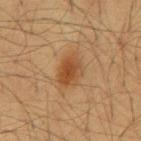Assessment: Imaged during a routine full-body skin examination; the lesion was not biopsied and no histopathology is available. Context: Approximately 4 mm at its widest. Automated tile analysis of the lesion measured a lesion area of about 8 mm², an eccentricity of roughly 0.75, and a symmetry-axis asymmetry near 0.2. And it measured an average lesion color of about L≈44 a*≈20 b*≈35 (CIELAB), roughly 9 lightness units darker than nearby skin, and a normalized border contrast of about 7.5. The analysis additionally found a border-irregularity index near 2/10 and radial color variation of about 1. The software also gave an automated nevus-likeness rating near 100 out of 100 and a detector confidence of about 100 out of 100 that the crop contains a lesion. Cropped from a whole-body photographic skin survey; the tile spans about 15 mm. Located on the abdomen. The patient is a male approximately 60 years of age.